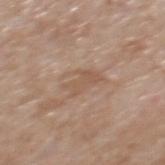Acquisition and patient details: The lesion is on the mid back. A region of skin cropped from a whole-body photographic capture, roughly 15 mm wide. A male patient aged approximately 65.